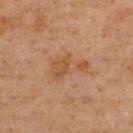Assessment:
The lesion was photographed on a routine skin check and not biopsied; there is no pathology result.
Acquisition and patient details:
Located on the back. The lesion's longest dimension is about 5 mm. A close-up tile cropped from a whole-body skin photograph, about 15 mm across. The patient is a male aged 53–57. The tile uses cross-polarized illumination.